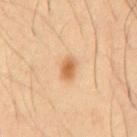Recorded during total-body skin imaging; not selected for excision or biopsy. An algorithmic analysis of the crop reported a lesion area of about 3.5 mm² and an outline eccentricity of about 0.75 (0 = round, 1 = elongated). The analysis additionally found border irregularity of about 1.5 on a 0–10 scale, internal color variation of about 2.5 on a 0–10 scale, and a peripheral color-asymmetry measure near 0.5. The software also gave a classifier nevus-likeness of about 95/100. The recorded lesion diameter is about 2.5 mm. The lesion is located on the front of the torso. A 15 mm crop from a total-body photograph taken for skin-cancer surveillance. Captured under cross-polarized illumination. The subject is a male in their 60s.